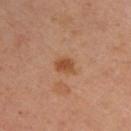<record>
<biopsy_status>not biopsied; imaged during a skin examination</biopsy_status>
<site>upper back</site>
<image>
  <source>total-body photography crop</source>
  <field_of_view_mm>15</field_of_view_mm>
</image>
<lesion_size>
  <long_diameter_mm_approx>2.5</long_diameter_mm_approx>
</lesion_size>
<patient>
  <sex>female</sex>
  <age_approx>45</age_approx>
</patient>
</record>